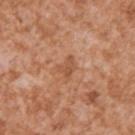The lesion was tiled from a total-body skin photograph and was not biopsied. Imaged with white-light lighting. Automated image analysis of the tile measured an area of roughly 3.5 mm², an eccentricity of roughly 0.65, and a shape-asymmetry score of about 0.5 (0 = symmetric). The software also gave roughly 8 lightness units darker than nearby skin and a normalized lesion–skin contrast near 6. On the arm. A male subject in their mid- to late 40s. Measured at roughly 2.5 mm in maximum diameter. Cropped from a total-body skin-imaging series; the visible field is about 15 mm.